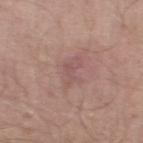workup — no biopsy performed (imaged during a skin exam)
imaging modality — ~15 mm crop, total-body skin-cancer survey
lesion size — about 4 mm
subject — male, about 50 years old
automated metrics — an area of roughly 6 mm² and an outline eccentricity of about 0.85 (0 = round, 1 = elongated); an average lesion color of about L≈53 a*≈20 b*≈21 (CIELAB) and roughly 6 lightness units darker than nearby skin
tile lighting — white-light
site — the left thigh